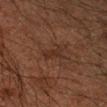Q: Lesion size?
A: about 2.5 mm
Q: Who is the patient?
A: male, aged 48–52
Q: What lighting was used for the tile?
A: cross-polarized illumination
Q: What did automated image analysis measure?
A: a lesion color around L≈22 a*≈15 b*≈20 in CIELAB, roughly 4 lightness units darker than nearby skin, and a normalized lesion–skin contrast near 5.5; an automated nevus-likeness rating near 0 out of 100 and a lesion-detection confidence of about 100/100
Q: What is the anatomic site?
A: the right forearm
Q: How was this image acquired?
A: total-body-photography crop, ~15 mm field of view